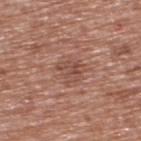Q: What is the imaging modality?
A: ~15 mm tile from a whole-body skin photo
Q: Who is the patient?
A: male, aged 73–77
Q: Lesion size?
A: ≈3 mm
Q: Where on the body is the lesion?
A: the back
Q: What lighting was used for the tile?
A: white-light